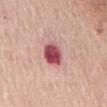Case summary:
- notes · catalogued during a skin exam; not biopsied
- patient · female, aged 63 to 67
- illumination · white-light
- image-analysis metrics · an average lesion color of about L≈53 a*≈32 b*≈20 (CIELAB) and a normalized lesion–skin contrast near 12
- site · the chest
- imaging modality · 15 mm crop, total-body photography